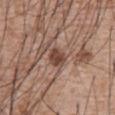The lesion was tiled from a total-body skin photograph and was not biopsied.
Automated image analysis of the tile measured a footprint of about 5 mm² and an outline eccentricity of about 0.6 (0 = round, 1 = elongated). The software also gave a classifier nevus-likeness of about 85/100 and a lesion-detection confidence of about 100/100.
Approximately 2.5 mm at its widest.
Imaged with white-light lighting.
The subject is a male about 60 years old.
A close-up tile cropped from a whole-body skin photograph, about 15 mm across.
The lesion is located on the front of the torso.